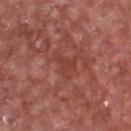{"biopsy_status": "not biopsied; imaged during a skin examination", "image": {"source": "total-body photography crop", "field_of_view_mm": 15}, "lesion_size": {"long_diameter_mm_approx": 3.0}, "automated_metrics": {"area_mm2_approx": 3.5, "eccentricity": 0.75, "border_irregularity_0_10": 6.0, "color_variation_0_10": 0.5, "peripheral_color_asymmetry": 0.0, "nevus_likeness_0_100": 0, "lesion_detection_confidence_0_100": 100}, "lighting": "white-light", "patient": {"sex": "male", "age_approx": 65}, "site": "chest"}